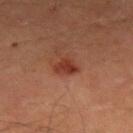Captured during whole-body skin photography for melanoma surveillance; the lesion was not biopsied. This image is a 15 mm lesion crop taken from a total-body photograph. The subject is a male in their mid- to late 60s. Located on the abdomen. Longest diameter approximately 2.5 mm. Imaged with cross-polarized lighting.